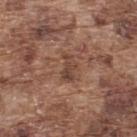Clinical impression: Captured during whole-body skin photography for melanoma surveillance; the lesion was not biopsied. Acquisition and patient details: A roughly 15 mm field-of-view crop from a total-body skin photograph. The lesion is located on the upper back. Measured at roughly 3.5 mm in maximum diameter. A male patient aged 73–77. Captured under white-light illumination.